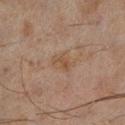{"biopsy_status": "not biopsied; imaged during a skin examination", "site": "left lower leg", "automated_metrics": {"area_mm2_approx": 4.5, "eccentricity": 0.65}, "lesion_size": {"long_diameter_mm_approx": 2.5}, "image": {"source": "total-body photography crop", "field_of_view_mm": 15}, "patient": {"sex": "male", "age_approx": 45}}